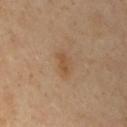Case summary:
* workup — no biopsy performed (imaged during a skin exam)